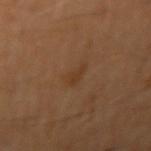This lesion was catalogued during total-body skin photography and was not selected for biopsy.
A male patient in their mid- to late 40s.
Located on the right upper arm.
A lesion tile, about 15 mm wide, cut from a 3D total-body photograph.